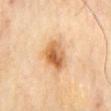{"biopsy_status": "not biopsied; imaged during a skin examination", "image": {"source": "total-body photography crop", "field_of_view_mm": 15}, "lighting": "cross-polarized", "patient": {"sex": "male", "age_approx": 65}, "site": "mid back", "lesion_size": {"long_diameter_mm_approx": 4.0}}